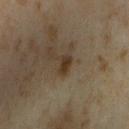Q: Was this lesion biopsied?
A: total-body-photography surveillance lesion; no biopsy
Q: What is the anatomic site?
A: the arm
Q: Who is the patient?
A: female, approximately 35 years of age
Q: What did automated image analysis measure?
A: a shape eccentricity near 0.8 and a symmetry-axis asymmetry near 0.35; a mean CIELAB color near L≈33 a*≈13 b*≈26, roughly 9 lightness units darker than nearby skin, and a normalized lesion–skin contrast near 9; a border-irregularity index near 3/10 and peripheral color asymmetry of about 1; an automated nevus-likeness rating near 45 out of 100 and a detector confidence of about 100 out of 100 that the crop contains a lesion
Q: What is the imaging modality?
A: ~15 mm crop, total-body skin-cancer survey
Q: What lighting was used for the tile?
A: cross-polarized illumination